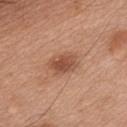Part of a total-body skin-imaging series; this lesion was reviewed on a skin check and was not flagged for biopsy. A roughly 15 mm field-of-view crop from a total-body skin photograph. The patient is a male aged approximately 45. The lesion-visualizer software estimated a lesion area of about 7.5 mm² and an eccentricity of roughly 0.75. And it measured about 11 CIELAB-L* units darker than the surrounding skin and a normalized lesion–skin contrast near 7.5. The analysis additionally found a border-irregularity rating of about 2.5/10 and internal color variation of about 3.5 on a 0–10 scale. The analysis additionally found a lesion-detection confidence of about 100/100. The lesion's longest dimension is about 3.5 mm. On the front of the torso. The tile uses white-light illumination.